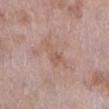Q: Was this lesion biopsied?
A: no biopsy performed (imaged during a skin exam)
Q: What did automated image analysis measure?
A: a footprint of about 6 mm² and a shape eccentricity near 0.9; a mean CIELAB color near L≈57 a*≈18 b*≈27, a lesion–skin lightness drop of about 6, and a normalized border contrast of about 5; a nevus-likeness score of about 0/100
Q: What is the imaging modality?
A: total-body-photography crop, ~15 mm field of view
Q: What is the anatomic site?
A: the left lower leg
Q: What are the patient's age and sex?
A: female, aged around 70